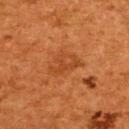Impression:
Captured during whole-body skin photography for melanoma surveillance; the lesion was not biopsied.
Context:
The lesion is located on the upper back. The subject is a female in their 50s. Measured at roughly 4 mm in maximum diameter. The total-body-photography lesion software estimated a footprint of about 7 mm², an eccentricity of roughly 0.75, and a shape-asymmetry score of about 0.4 (0 = symmetric). The analysis additionally found about 6 CIELAB-L* units darker than the surrounding skin. And it measured a border-irregularity rating of about 5/10 and peripheral color asymmetry of about 1. And it measured an automated nevus-likeness rating near 0 out of 100 and lesion-presence confidence of about 100/100. A 15 mm close-up extracted from a 3D total-body photography capture.